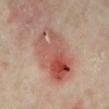Part of a total-body skin-imaging series; this lesion was reviewed on a skin check and was not flagged for biopsy. The lesion's longest dimension is about 8 mm. A region of skin cropped from a whole-body photographic capture, roughly 15 mm wide. Captured under cross-polarized illumination. A female subject, about 35 years old. Located on the left lower leg. Automated image analysis of the tile measured a footprint of about 26 mm², an outline eccentricity of about 0.85 (0 = round, 1 = elongated), and a symmetry-axis asymmetry near 0.15. And it measured a lesion color around L≈51 a*≈24 b*≈26 in CIELAB, a lesion–skin lightness drop of about 11, and a normalized lesion–skin contrast near 7.5. The analysis additionally found border irregularity of about 2.5 on a 0–10 scale, a color-variation rating of about 10/10, and peripheral color asymmetry of about 5.5. The software also gave a nevus-likeness score of about 0/100 and a lesion-detection confidence of about 100/100.